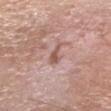Case summary:
* notes: total-body-photography surveillance lesion; no biopsy
* lesion diameter: ~3 mm (longest diameter)
* imaging modality: total-body-photography crop, ~15 mm field of view
* subject: male, aged around 70
* location: the head or neck
* lighting: white-light